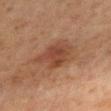Context: This is a cross-polarized tile. On the chest. Approximately 5 mm at its widest. A female subject, in their 60s. Cropped from a whole-body photographic skin survey; the tile spans about 15 mm.